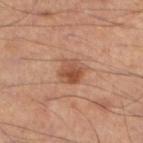Q: Was a biopsy performed?
A: imaged on a skin check; not biopsied
Q: Who is the patient?
A: male, roughly 50 years of age
Q: How large is the lesion?
A: ~3 mm (longest diameter)
Q: What lighting was used for the tile?
A: cross-polarized
Q: Where on the body is the lesion?
A: the left leg
Q: What kind of image is this?
A: ~15 mm crop, total-body skin-cancer survey